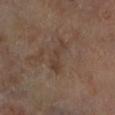Background: Automated image analysis of the tile measured two-axis asymmetry of about 0.5. The analysis additionally found an average lesion color of about L≈37 a*≈15 b*≈23 (CIELAB), a lesion–skin lightness drop of about 6, and a lesion-to-skin contrast of about 5.5 (normalized; higher = more distinct). The analysis additionally found a nevus-likeness score of about 0/100. A male patient, aged 68–72. From the left lower leg. A region of skin cropped from a whole-body photographic capture, roughly 15 mm wide. Longest diameter approximately 4.5 mm.